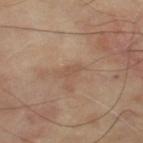Assessment:
Recorded during total-body skin imaging; not selected for excision or biopsy.
Clinical summary:
Located on the left thigh. Cropped from a total-body skin-imaging series; the visible field is about 15 mm. A male patient approximately 65 years of age. Automated image analysis of the tile measured a lesion–skin lightness drop of about 6 and a lesion-to-skin contrast of about 4.5 (normalized; higher = more distinct).